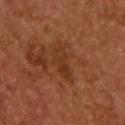This lesion was catalogued during total-body skin photography and was not selected for biopsy.
Located on the upper back.
The total-body-photography lesion software estimated a lesion area of about 7 mm², a shape eccentricity near 0.9, and a shape-asymmetry score of about 0.35 (0 = symmetric). And it measured an average lesion color of about L≈31 a*≈22 b*≈30 (CIELAB) and roughly 5 lightness units darker than nearby skin. The software also gave a border-irregularity index near 4.5/10, a within-lesion color-variation index near 3.5/10, and a peripheral color-asymmetry measure near 1.5. And it measured an automated nevus-likeness rating near 0 out of 100 and a lesion-detection confidence of about 100/100.
The lesion's longest dimension is about 4.5 mm.
A close-up tile cropped from a whole-body skin photograph, about 15 mm across.
A male patient, in their mid- to late 50s.